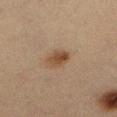Q: Was a biopsy performed?
A: no biopsy performed (imaged during a skin exam)